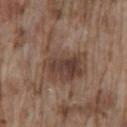Imaged during a routine full-body skin examination; the lesion was not biopsied and no histopathology is available. A 15 mm close-up extracted from a 3D total-body photography capture. Captured under white-light illumination. The lesion is on the lower back. The subject is a male approximately 75 years of age.